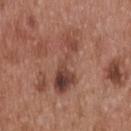Q: Was this lesion biopsied?
A: imaged on a skin check; not biopsied
Q: What is the lesion's diameter?
A: about 6.5 mm
Q: How was this image acquired?
A: ~15 mm tile from a whole-body skin photo
Q: What is the anatomic site?
A: the upper back
Q: What are the patient's age and sex?
A: male, in their mid-50s